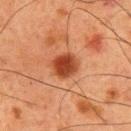automated metrics: an area of roughly 8 mm² and two-axis asymmetry of about 0.15
patient: male, about 60 years old
image: ~15 mm crop, total-body skin-cancer survey
illumination: cross-polarized illumination
diameter: about 3.5 mm
body site: the upper back